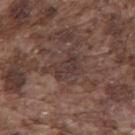{
  "lesion_size": {
    "long_diameter_mm_approx": 3.5
  },
  "image": {
    "source": "total-body photography crop",
    "field_of_view_mm": 15
  },
  "lighting": "white-light",
  "automated_metrics": {
    "area_mm2_approx": 6.0,
    "shape_asymmetry": 0.3,
    "vs_skin_darker_L": 6.0,
    "vs_skin_contrast_norm": 6.5,
    "border_irregularity_0_10": 3.0,
    "color_variation_0_10": 3.0,
    "peripheral_color_asymmetry": 1.0
  },
  "site": "mid back",
  "patient": {
    "sex": "male",
    "age_approx": 75
  }
}